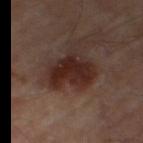Clinical impression: This lesion was catalogued during total-body skin photography and was not selected for biopsy. Background: A roughly 15 mm field-of-view crop from a total-body skin photograph. The tile uses cross-polarized illumination. About 5 mm across. On the right thigh.